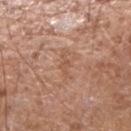Clinical impression: Part of a total-body skin-imaging series; this lesion was reviewed on a skin check and was not flagged for biopsy. Image and clinical context: On the right forearm. A male subject in their mid-70s. A close-up tile cropped from a whole-body skin photograph, about 15 mm across.